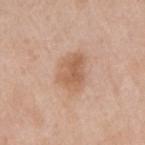Clinical impression:
The lesion was tiled from a total-body skin photograph and was not biopsied.
Clinical summary:
Approximately 4.5 mm at its widest. Captured under white-light illumination. From the right upper arm. A region of skin cropped from a whole-body photographic capture, roughly 15 mm wide. The total-body-photography lesion software estimated a lesion area of about 11 mm², an eccentricity of roughly 0.7, and a symmetry-axis asymmetry near 0.25. It also reported a border-irregularity index near 2.5/10 and a peripheral color-asymmetry measure near 1.5. The patient is a female in their 40s.